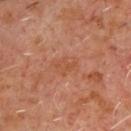| key | value |
|---|---|
| site | the chest |
| size | ~3 mm (longest diameter) |
| patient | male, approximately 60 years of age |
| image source | 15 mm crop, total-body photography |
| tile lighting | cross-polarized illumination |
| TBP lesion metrics | a lesion–skin lightness drop of about 5 and a normalized lesion–skin contrast near 4.5; internal color variation of about 2 on a 0–10 scale and radial color variation of about 1 |